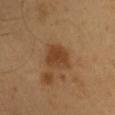About 3.5 mm across. Automated image analysis of the tile measured a lesion color around L≈39 a*≈20 b*≈33 in CIELAB, a lesion–skin lightness drop of about 9, and a lesion-to-skin contrast of about 8 (normalized; higher = more distinct). And it measured border irregularity of about 2.5 on a 0–10 scale and internal color variation of about 2 on a 0–10 scale. The software also gave an automated nevus-likeness rating near 95 out of 100 and lesion-presence confidence of about 100/100. A 15 mm close-up tile from a total-body photography series done for melanoma screening. A male patient approximately 55 years of age. The lesion is on the front of the torso.